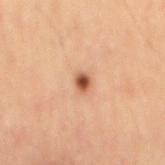<record>
<image>
  <source>total-body photography crop</source>
  <field_of_view_mm>15</field_of_view_mm>
</image>
<site>mid back</site>
<lighting>cross-polarized</lighting>
<patient>
  <sex>male</sex>
  <age_approx>55</age_approx>
</patient>
</record>